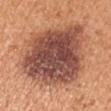workup: total-body-photography surveillance lesion; no biopsy | image source: 15 mm crop, total-body photography | illumination: white-light illumination | diameter: ~10 mm (longest diameter) | body site: the right upper arm | patient: male, aged 53–57 | automated metrics: a lesion area of about 55 mm², a shape eccentricity near 0.6, and two-axis asymmetry of about 0.2; a mean CIELAB color near L≈49 a*≈24 b*≈28 and a lesion–skin lightness drop of about 16; a border-irregularity rating of about 2.5/10, internal color variation of about 7 on a 0–10 scale, and a peripheral color-asymmetry measure near 2.